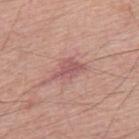The lesion was photographed on a routine skin check and not biopsied; there is no pathology result. Longest diameter approximately 4 mm. A 15 mm close-up extracted from a 3D total-body photography capture. The total-body-photography lesion software estimated a border-irregularity rating of about 4/10 and a within-lesion color-variation index near 2/10. The lesion is located on the mid back. This is a white-light tile. A male subject, approximately 60 years of age.